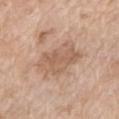Findings:
* notes: no biopsy performed (imaged during a skin exam)
* site: the left upper arm
* image-analysis metrics: a lesion area of about 16 mm², a shape eccentricity near 0.75, and a shape-asymmetry score of about 0.2 (0 = symmetric); a lesion color around L≈59 a*≈18 b*≈30 in CIELAB, about 9 CIELAB-L* units darker than the surrounding skin, and a lesion-to-skin contrast of about 6 (normalized; higher = more distinct)
* size: about 5.5 mm
* tile lighting: white-light
* subject: female, roughly 75 years of age
* imaging modality: ~15 mm tile from a whole-body skin photo